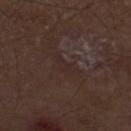anatomic site: the left forearm; patient: male, aged 63 to 67; acquisition: ~15 mm crop, total-body skin-cancer survey; diameter: ~3.5 mm (longest diameter); histopathologic diagnosis: an actinic keratosis — a borderline lesion of uncertain malignant potential.